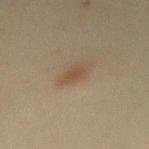biopsy status: no biopsy performed (imaged during a skin exam).